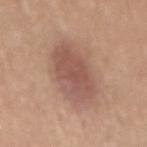The lesion is on the back. Longest diameter approximately 6.5 mm. This image is a 15 mm lesion crop taken from a total-body photograph. This is a white-light tile. The subject is a female approximately 55 years of age.